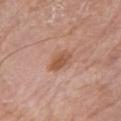The lesion was tiled from a total-body skin photograph and was not biopsied.
Cropped from a whole-body photographic skin survey; the tile spans about 15 mm.
On the left upper arm.
The lesion-visualizer software estimated an area of roughly 5 mm² and an eccentricity of roughly 0.8. The analysis additionally found a lesion-detection confidence of about 100/100.
A male patient, in their 80s.
Imaged with white-light lighting.
The lesion's longest dimension is about 3.5 mm.